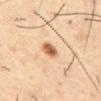A 15 mm close-up tile from a total-body photography series done for melanoma screening. The patient is a male aged approximately 55. Approximately 3 mm at its widest. Automated image analysis of the tile measured an average lesion color of about L≈61 a*≈21 b*≈36 (CIELAB), about 16 CIELAB-L* units darker than the surrounding skin, and a normalized lesion–skin contrast near 9.5. The software also gave border irregularity of about 2 on a 0–10 scale, a color-variation rating of about 4.5/10, and a peripheral color-asymmetry measure near 1.5. And it measured a nevus-likeness score of about 100/100 and lesion-presence confidence of about 100/100. On the abdomen.